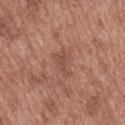Q: Is there a histopathology result?
A: total-body-photography surveillance lesion; no biopsy
Q: What is the imaging modality?
A: ~15 mm crop, total-body skin-cancer survey
Q: Who is the patient?
A: male, aged around 50
Q: Lesion location?
A: the mid back